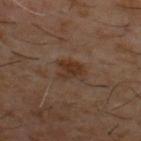notes — catalogued during a skin exam; not biopsied | subject — male, roughly 60 years of age | site — the back | image — ~15 mm crop, total-body skin-cancer survey.